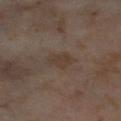No biopsy was performed on this lesion — it was imaged during a full skin examination and was not determined to be concerning. A female subject aged approximately 55. About 3 mm across. Captured under cross-polarized illumination. Located on the left thigh. A 15 mm close-up extracted from a 3D total-body photography capture. The total-body-photography lesion software estimated a footprint of about 5.5 mm², an eccentricity of roughly 0.65, and a symmetry-axis asymmetry near 0.25. The software also gave a mean CIELAB color near L≈38 a*≈13 b*≈23 and a normalized lesion–skin contrast near 5.5. The software also gave a within-lesion color-variation index near 2/10 and a peripheral color-asymmetry measure near 1. And it measured a nevus-likeness score of about 0/100 and a lesion-detection confidence of about 100/100.